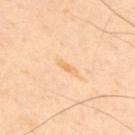– follow-up · imaged on a skin check; not biopsied
– body site · the back
– lesion size · ≈3 mm
– illumination · cross-polarized illumination
– automated lesion analysis · a lesion color around L≈74 a*≈19 b*≈42 in CIELAB, a lesion–skin lightness drop of about 6, and a normalized lesion–skin contrast near 6; a border-irregularity index near 4/10, internal color variation of about 0 on a 0–10 scale, and radial color variation of about 0; a detector confidence of about 100 out of 100 that the crop contains a lesion
– subject · male, aged approximately 45
– imaging modality · ~15 mm crop, total-body skin-cancer survey